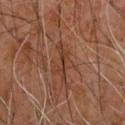<tbp_lesion>
  <biopsy_status>not biopsied; imaged during a skin examination</biopsy_status>
  <site>upper back</site>
  <patient>
    <sex>male</sex>
    <age_approx>60</age_approx>
  </patient>
  <lesion_size>
    <long_diameter_mm_approx>4.5</long_diameter_mm_approx>
  </lesion_size>
  <lighting>cross-polarized</lighting>
  <automated_metrics>
    <area_mm2_approx>6.5</area_mm2_approx>
    <eccentricity>0.9</eccentricity>
    <shape_asymmetry>0.55</shape_asymmetry>
    <vs_skin_darker_L>6.0</vs_skin_darker_L>
    <vs_skin_contrast_norm>6.0</vs_skin_contrast_norm>
    <nevus_likeness_0_100>0</nevus_likeness_0_100>
    <lesion_detection_confidence_0_100>65</lesion_detection_confidence_0_100>
  </automated_metrics>
  <image>
    <source>total-body photography crop</source>
    <field_of_view_mm>15</field_of_view_mm>
  </image>
</tbp_lesion>